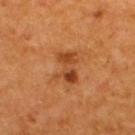Imaged during a routine full-body skin examination; the lesion was not biopsied and no histopathology is available.
The tile uses cross-polarized illumination.
The lesion is on the back.
A roughly 15 mm field-of-view crop from a total-body skin photograph.
About 4 mm across.
The patient is a male aged 53 to 57.